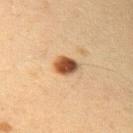{"biopsy_status": "not biopsied; imaged during a skin examination", "patient": {"sex": "female", "age_approx": 40}, "site": "arm", "lighting": "cross-polarized", "image": {"source": "total-body photography crop", "field_of_view_mm": 15}}